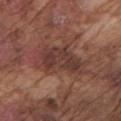Impression:
No biopsy was performed on this lesion — it was imaged during a full skin examination and was not determined to be concerning.
Clinical summary:
A male subject aged around 75. A 15 mm crop from a total-body photograph taken for skin-cancer surveillance. Imaged with white-light lighting. Located on the left upper arm.